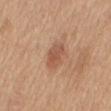Assessment:
Captured during whole-body skin photography for melanoma surveillance; the lesion was not biopsied.
Context:
The subject is a female roughly 55 years of age. The total-body-photography lesion software estimated an eccentricity of roughly 0.8. And it measured border irregularity of about 2.5 on a 0–10 scale, a within-lesion color-variation index near 3/10, and peripheral color asymmetry of about 1. A 15 mm close-up tile from a total-body photography series done for melanoma screening. This is a white-light tile. The lesion is located on the mid back.